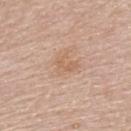Captured during whole-body skin photography for melanoma surveillance; the lesion was not biopsied. A female patient aged around 65. On the upper back. The tile uses white-light illumination. Automated tile analysis of the lesion measured a shape eccentricity near 0.75 and a symmetry-axis asymmetry near 0.6. The analysis additionally found a lesion-to-skin contrast of about 5 (normalized; higher = more distinct). The analysis additionally found a border-irregularity index near 6.5/10, internal color variation of about 0.5 on a 0–10 scale, and a peripheral color-asymmetry measure near 0. And it measured a classifier nevus-likeness of about 0/100 and lesion-presence confidence of about 100/100. A region of skin cropped from a whole-body photographic capture, roughly 15 mm wide.